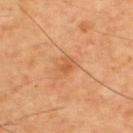The lesion was photographed on a routine skin check and not biopsied; there is no pathology result.
The lesion is on the upper back.
The patient is a male aged 58 to 62.
Measured at roughly 2.5 mm in maximum diameter.
The total-body-photography lesion software estimated an area of roughly 4 mm², an eccentricity of roughly 0.65, and two-axis asymmetry of about 0.3. It also reported a mean CIELAB color near L≈56 a*≈25 b*≈40, roughly 7 lightness units darker than nearby skin, and a lesion-to-skin contrast of about 5 (normalized; higher = more distinct). The software also gave border irregularity of about 3 on a 0–10 scale and a color-variation rating of about 2.5/10. The software also gave a nevus-likeness score of about 5/100 and lesion-presence confidence of about 100/100.
The tile uses cross-polarized illumination.
A 15 mm close-up tile from a total-body photography series done for melanoma screening.